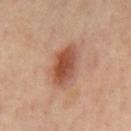{
  "site": "back",
  "image": {
    "source": "total-body photography crop",
    "field_of_view_mm": 15
  },
  "patient": {
    "sex": "male",
    "age_approx": 65
  },
  "lighting": "cross-polarized",
  "lesion_size": {
    "long_diameter_mm_approx": 5.0
  }
}